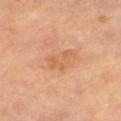Part of a total-body skin-imaging series; this lesion was reviewed on a skin check and was not flagged for biopsy. This is a cross-polarized tile. Measured at roughly 3.5 mm in maximum diameter. The lesion is on the right leg. A region of skin cropped from a whole-body photographic capture, roughly 15 mm wide. A female subject aged around 60. The total-body-photography lesion software estimated a lesion area of about 6.5 mm². The analysis additionally found an average lesion color of about L≈63 a*≈25 b*≈39 (CIELAB) and a lesion–skin lightness drop of about 7. It also reported border irregularity of about 3.5 on a 0–10 scale, a within-lesion color-variation index near 3/10, and peripheral color asymmetry of about 1. It also reported an automated nevus-likeness rating near 0 out of 100 and lesion-presence confidence of about 100/100.